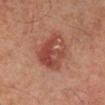imaging modality: ~15 mm tile from a whole-body skin photo
patient: male, aged 48 to 52
anatomic site: the left leg